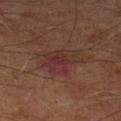Clinical summary:
A close-up tile cropped from a whole-body skin photograph, about 15 mm across. About 5 mm across. A male subject in their mid- to late 60s. Captured under cross-polarized illumination.